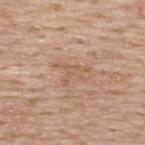Case summary:
– workup: imaged on a skin check; not biopsied